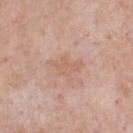Imaged during a routine full-body skin examination; the lesion was not biopsied and no histopathology is available.
The lesion is located on the chest.
An algorithmic analysis of the crop reported a footprint of about 6 mm², an eccentricity of roughly 0.8, and two-axis asymmetry of about 0.4. The analysis additionally found a mean CIELAB color near L≈62 a*≈20 b*≈29, about 6 CIELAB-L* units darker than the surrounding skin, and a normalized lesion–skin contrast near 5.
About 4 mm across.
A lesion tile, about 15 mm wide, cut from a 3D total-body photograph.
A male subject, about 55 years old.